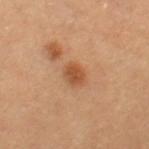Q: Was a biopsy performed?
A: catalogued during a skin exam; not biopsied
Q: How was the tile lit?
A: cross-polarized
Q: What kind of image is this?
A: 15 mm crop, total-body photography
Q: What are the patient's age and sex?
A: female, aged approximately 60
Q: Automated lesion metrics?
A: a mean CIELAB color near L≈53 a*≈25 b*≈38, about 10 CIELAB-L* units darker than the surrounding skin, and a lesion-to-skin contrast of about 7.5 (normalized; higher = more distinct)
Q: Where on the body is the lesion?
A: the leg